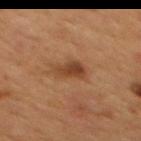{
  "biopsy_status": "not biopsied; imaged during a skin examination",
  "patient": {
    "sex": "male",
    "age_approx": 55
  },
  "site": "mid back",
  "image": {
    "source": "total-body photography crop",
    "field_of_view_mm": 15
  },
  "lesion_size": {
    "long_diameter_mm_approx": 3.0
  },
  "lighting": "cross-polarized"
}